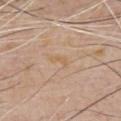Impression: Part of a total-body skin-imaging series; this lesion was reviewed on a skin check and was not flagged for biopsy. Background: Captured under white-light illumination. The lesion is located on the chest. Automated image analysis of the tile measured a border-irregularity rating of about 4.5/10. A 15 mm close-up extracted from a 3D total-body photography capture. The lesion's longest dimension is about 2.5 mm. A male subject, aged approximately 55.